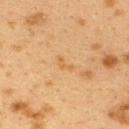Clinical impression:
This lesion was catalogued during total-body skin photography and was not selected for biopsy.
Background:
The patient is a female aged 38 to 42. This is a cross-polarized tile. A close-up tile cropped from a whole-body skin photograph, about 15 mm across. The lesion is on the upper back.